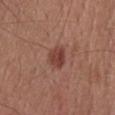Clinical impression:
Imaged during a routine full-body skin examination; the lesion was not biopsied and no histopathology is available.
Clinical summary:
A female subject aged 38 to 42. The lesion-visualizer software estimated a footprint of about 5.5 mm², an outline eccentricity of about 0.5 (0 = round, 1 = elongated), and two-axis asymmetry of about 0.25. It also reported a border-irregularity rating of about 2/10, a color-variation rating of about 3.5/10, and peripheral color asymmetry of about 1. From the head or neck. Imaged with white-light lighting. A 15 mm crop from a total-body photograph taken for skin-cancer surveillance. The recorded lesion diameter is about 3 mm.